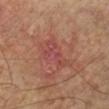Assessment:
Recorded during total-body skin imaging; not selected for excision or biopsy.
Image and clinical context:
A lesion tile, about 15 mm wide, cut from a 3D total-body photograph. A male subject in their mid- to late 50s. Imaged with cross-polarized lighting. On the leg.